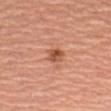Image and clinical context:
Measured at roughly 3 mm in maximum diameter. Automated image analysis of the tile measured a within-lesion color-variation index near 4.5/10 and a peripheral color-asymmetry measure near 1.5. The software also gave a nevus-likeness score of about 85/100 and a lesion-detection confidence of about 100/100. The subject is a female aged around 55. From the left upper arm. A region of skin cropped from a whole-body photographic capture, roughly 15 mm wide.